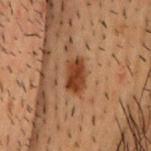Context:
The lesion-visualizer software estimated a shape eccentricity near 0.8 and two-axis asymmetry of about 0.2. A 15 mm close-up tile from a total-body photography series done for melanoma screening. A male subject in their 50s. About 3.5 mm across. From the head or neck.